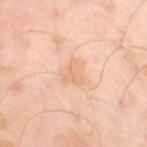Clinical impression: Captured during whole-body skin photography for melanoma surveillance; the lesion was not biopsied. Clinical summary: Captured under cross-polarized illumination. The lesion's longest dimension is about 3 mm. A male subject aged approximately 50. A roughly 15 mm field-of-view crop from a total-body skin photograph. The lesion is located on the left leg. The lesion-visualizer software estimated a lesion area of about 6 mm² and two-axis asymmetry of about 0.4. The software also gave about 6 CIELAB-L* units darker than the surrounding skin and a lesion-to-skin contrast of about 4.5 (normalized; higher = more distinct). And it measured a border-irregularity rating of about 4/10 and internal color variation of about 2.5 on a 0–10 scale.